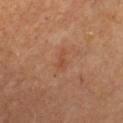Recorded during total-body skin imaging; not selected for excision or biopsy.
Located on the chest.
About 2.5 mm across.
Captured under cross-polarized illumination.
A roughly 15 mm field-of-view crop from a total-body skin photograph.
The patient is aged 58–62.